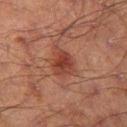Findings:
– tile lighting — cross-polarized
– image source — ~15 mm crop, total-body skin-cancer survey
– subject — male, aged 68 to 72
– anatomic site — the leg
– lesion diameter — ≈3.5 mm